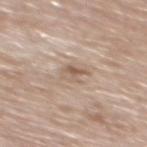Part of a total-body skin-imaging series; this lesion was reviewed on a skin check and was not flagged for biopsy.
A male patient roughly 70 years of age.
Located on the upper back.
Cropped from a total-body skin-imaging series; the visible field is about 15 mm.
This is a white-light tile.
Approximately 3 mm at its widest.
The lesion-visualizer software estimated an area of roughly 4.5 mm² and a symmetry-axis asymmetry near 0.35. It also reported a mean CIELAB color near L≈59 a*≈14 b*≈27, roughly 9 lightness units darker than nearby skin, and a normalized lesion–skin contrast near 6.5.